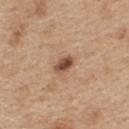The lesion was photographed on a routine skin check and not biopsied; there is no pathology result.
Captured under white-light illumination.
On the upper back.
A female subject roughly 45 years of age.
Automated tile analysis of the lesion measured an area of roughly 3.5 mm², an outline eccentricity of about 0.8 (0 = round, 1 = elongated), and a shape-asymmetry score of about 0.25 (0 = symmetric).
Cropped from a total-body skin-imaging series; the visible field is about 15 mm.
The lesion's longest dimension is about 2.5 mm.